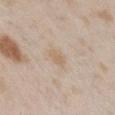Clinical impression: Imaged during a routine full-body skin examination; the lesion was not biopsied and no histopathology is available. Background: This is a white-light tile. The lesion is on the chest. A 15 mm close-up tile from a total-body photography series done for melanoma screening. A female subject, in their mid- to late 20s.